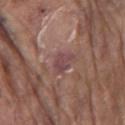Impression:
Recorded during total-body skin imaging; not selected for excision or biopsy.
Acquisition and patient details:
Located on the right upper arm. The lesion-visualizer software estimated an area of roughly 5 mm², an eccentricity of roughly 0.55, and a symmetry-axis asymmetry near 0.3. The analysis additionally found a mean CIELAB color near L≈45 a*≈21 b*≈17, roughly 7 lightness units darker than nearby skin, and a normalized lesion–skin contrast near 7.5. The analysis additionally found an automated nevus-likeness rating near 0 out of 100 and lesion-presence confidence of about 95/100. Cropped from a whole-body photographic skin survey; the tile spans about 15 mm. A male patient, approximately 80 years of age.